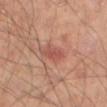The lesion was photographed on a routine skin check and not biopsied; there is no pathology result. Longest diameter approximately 2.5 mm. A male patient aged 63–67. Captured under cross-polarized illumination. The lesion is located on the left thigh. A close-up tile cropped from a whole-body skin photograph, about 15 mm across.